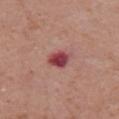{"biopsy_status": "not biopsied; imaged during a skin examination", "image": {"source": "total-body photography crop", "field_of_view_mm": 15}, "lighting": "white-light", "automated_metrics": {"area_mm2_approx": 5.5, "border_irregularity_0_10": 2.5, "peripheral_color_asymmetry": 1.5, "nevus_likeness_0_100": 0, "lesion_detection_confidence_0_100": 100}, "patient": {"sex": "male", "age_approx": 70}, "site": "chest", "lesion_size": {"long_diameter_mm_approx": 2.5}}